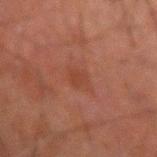{"biopsy_status": "not biopsied; imaged during a skin examination", "lesion_size": {"long_diameter_mm_approx": 3.0}, "patient": {"sex": "male", "age_approx": 60}, "site": "left forearm", "image": {"source": "total-body photography crop", "field_of_view_mm": 15}, "lighting": "cross-polarized"}